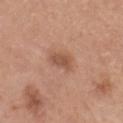<case>
  <biopsy_status>not biopsied; imaged during a skin examination</biopsy_status>
  <image>
    <source>total-body photography crop</source>
    <field_of_view_mm>15</field_of_view_mm>
  </image>
  <patient>
    <sex>male</sex>
    <age_approx>35</age_approx>
  </patient>
  <lesion_size>
    <long_diameter_mm_approx>3.0</long_diameter_mm_approx>
  </lesion_size>
  <site>head or neck</site>
  <lighting>white-light</lighting>
</case>